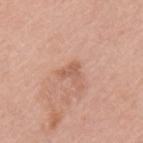Q: Was a biopsy performed?
A: imaged on a skin check; not biopsied
Q: What is the lesion's diameter?
A: ≈2.5 mm
Q: What is the imaging modality?
A: ~15 mm tile from a whole-body skin photo
Q: Automated lesion metrics?
A: an area of roughly 3.5 mm², an eccentricity of roughly 0.7, and a symmetry-axis asymmetry near 0.55; an average lesion color of about L≈59 a*≈23 b*≈31 (CIELAB) and a lesion-to-skin contrast of about 5.5 (normalized; higher = more distinct); a peripheral color-asymmetry measure near 0; a nevus-likeness score of about 0/100 and a detector confidence of about 100 out of 100 that the crop contains a lesion
Q: What are the patient's age and sex?
A: female, aged 58 to 62
Q: Lesion location?
A: the upper back